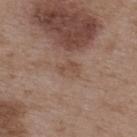| feature | finding |
|---|---|
| biopsy status | imaged on a skin check; not biopsied |
| subject | male, about 50 years old |
| acquisition | ~15 mm tile from a whole-body skin photo |
| illumination | white-light |
| location | the upper back |